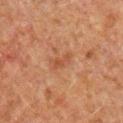notes=catalogued during a skin exam; not biopsied
patient=male, roughly 60 years of age
site=the chest
imaging modality=15 mm crop, total-body photography
tile lighting=cross-polarized illumination
lesion diameter=≈2.5 mm
automated metrics=a lesion color around L≈38 a*≈20 b*≈29 in CIELAB and a normalized border contrast of about 5.5